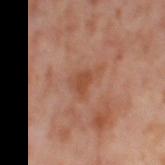Clinical impression:
Part of a total-body skin-imaging series; this lesion was reviewed on a skin check and was not flagged for biopsy.
Clinical summary:
The tile uses cross-polarized illumination. Automated image analysis of the tile measured an outline eccentricity of about 0.75 (0 = round, 1 = elongated) and a shape-asymmetry score of about 0.35 (0 = symmetric). It also reported a lesion color around L≈49 a*≈24 b*≈33 in CIELAB and a lesion-to-skin contrast of about 7 (normalized; higher = more distinct). And it measured a border-irregularity index near 3/10 and radial color variation of about 0.5. The software also gave a classifier nevus-likeness of about 0/100. From the left thigh. Cropped from a total-body skin-imaging series; the visible field is about 15 mm. The subject is a female aged around 55.